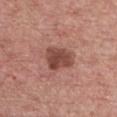Recorded during total-body skin imaging; not selected for excision or biopsy. Cropped from a whole-body photographic skin survey; the tile spans about 15 mm. Longest diameter approximately 3.5 mm. On the chest. Imaged with white-light lighting. A male subject aged 58 to 62.